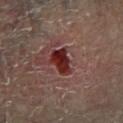| field | value |
|---|---|
| notes | no biopsy performed (imaged during a skin exam) |
| patient | male, aged around 85 or older |
| imaging modality | 15 mm crop, total-body photography |
| lesion size | about 3.5 mm |
| location | the right lower leg |
| lighting | cross-polarized illumination |